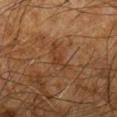Clinical impression: Part of a total-body skin-imaging series; this lesion was reviewed on a skin check and was not flagged for biopsy. Background: The tile uses cross-polarized illumination. About 3.5 mm across. Automated image analysis of the tile measured an area of roughly 4 mm², an eccentricity of roughly 0.8, and a shape-asymmetry score of about 0.45 (0 = symmetric). The software also gave a lesion color around L≈30 a*≈18 b*≈27 in CIELAB, roughly 5 lightness units darker than nearby skin, and a normalized lesion–skin contrast near 5. The software also gave a within-lesion color-variation index near 1.5/10 and radial color variation of about 0.5. And it measured an automated nevus-likeness rating near 0 out of 100 and a detector confidence of about 95 out of 100 that the crop contains a lesion. The lesion is on the left forearm. A region of skin cropped from a whole-body photographic capture, roughly 15 mm wide. The subject is a male aged around 65.